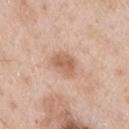Clinical impression:
No biopsy was performed on this lesion — it was imaged during a full skin examination and was not determined to be concerning.
Acquisition and patient details:
The tile uses white-light illumination. A lesion tile, about 15 mm wide, cut from a 3D total-body photograph. About 3.5 mm across. A male patient, in their mid- to late 50s. From the left upper arm.